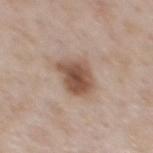Captured during whole-body skin photography for melanoma surveillance; the lesion was not biopsied.
The subject is a male in their 50s.
The tile uses white-light illumination.
A lesion tile, about 15 mm wide, cut from a 3D total-body photograph.
The lesion is located on the mid back.
About 4 mm across.